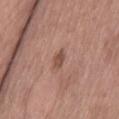{"biopsy_status": "not biopsied; imaged during a skin examination"}